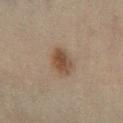Q: Was this lesion biopsied?
A: catalogued during a skin exam; not biopsied
Q: How large is the lesion?
A: ≈3.5 mm
Q: Patient demographics?
A: male, aged approximately 45
Q: What is the anatomic site?
A: the right lower leg
Q: How was this image acquired?
A: ~15 mm crop, total-body skin-cancer survey